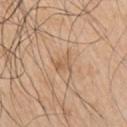biopsy status: imaged on a skin check; not biopsied | body site: the right upper arm | image source: ~15 mm crop, total-body skin-cancer survey | lighting: white-light | patient: male, roughly 75 years of age.